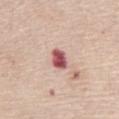The lesion was photographed on a routine skin check and not biopsied; there is no pathology result.
A female subject, approximately 70 years of age.
The lesion is on the chest.
Longest diameter approximately 2.5 mm.
Captured under white-light illumination.
Automated image analysis of the tile measured an average lesion color of about L≈55 a*≈29 b*≈22 (CIELAB) and a lesion–skin lightness drop of about 19.
A region of skin cropped from a whole-body photographic capture, roughly 15 mm wide.